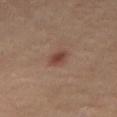notes — total-body-photography surveillance lesion; no biopsy | diameter — ~2.5 mm (longest diameter) | imaging modality — total-body-photography crop, ~15 mm field of view | location — the right thigh | lighting — cross-polarized illumination | patient — female, in their 40s.